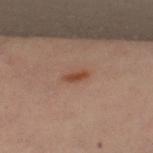Q: Is there a histopathology result?
A: total-body-photography surveillance lesion; no biopsy
Q: Who is the patient?
A: female, aged around 40
Q: How was this image acquired?
A: ~15 mm crop, total-body skin-cancer survey
Q: Lesion location?
A: the upper back